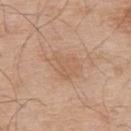Clinical impression:
Captured during whole-body skin photography for melanoma surveillance; the lesion was not biopsied.
Background:
The patient is a male aged around 55. A roughly 15 mm field-of-view crop from a total-body skin photograph. The lesion is located on the upper back.